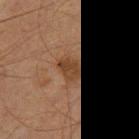The lesion was photographed on a routine skin check and not biopsied; there is no pathology result. The lesion's longest dimension is about 3 mm. Located on the right thigh. The subject is a male aged around 60. An algorithmic analysis of the crop reported a symmetry-axis asymmetry near 0.25. It also reported a mean CIELAB color near L≈34 a*≈17 b*≈27 and a normalized border contrast of about 7.5. It also reported a border-irregularity index near 2.5/10 and a within-lesion color-variation index near 2.5/10. A roughly 15 mm field-of-view crop from a total-body skin photograph.